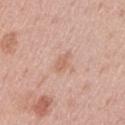Background:
This image is a 15 mm lesion crop taken from a total-body photograph. Automated tile analysis of the lesion measured a footprint of about 3 mm², an eccentricity of roughly 0.85, and a symmetry-axis asymmetry near 0.3. It also reported a lesion color around L≈63 a*≈20 b*≈30 in CIELAB, about 7 CIELAB-L* units darker than the surrounding skin, and a normalized border contrast of about 5.5. About 2.5 mm across. A male patient, about 45 years old. On the left upper arm.